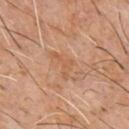workup = imaged on a skin check; not biopsied
patient = male, approximately 60 years of age
tile lighting = cross-polarized
image source = ~15 mm tile from a whole-body skin photo
TBP lesion metrics = a border-irregularity rating of about 7.5/10, a color-variation rating of about 0.5/10, and a peripheral color-asymmetry measure near 0; an automated nevus-likeness rating near 0 out of 100 and a lesion-detection confidence of about 100/100
diameter = ≈3.5 mm
body site = the front of the torso